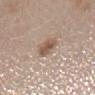Q: Was a biopsy performed?
A: catalogued during a skin exam; not biopsied
Q: What is the lesion's diameter?
A: ≈3 mm
Q: How was the tile lit?
A: white-light
Q: What is the imaging modality?
A: ~15 mm tile from a whole-body skin photo
Q: Lesion location?
A: the mid back
Q: What are the patient's age and sex?
A: female, about 45 years old